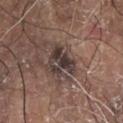Assessment: The lesion was tiled from a total-body skin photograph and was not biopsied. Background: Located on the chest. An algorithmic analysis of the crop reported a lesion area of about 8 mm², an eccentricity of roughly 0.65, and a shape-asymmetry score of about 0.45 (0 = symmetric). The analysis additionally found an average lesion color of about L≈36 a*≈12 b*≈17 (CIELAB), roughly 12 lightness units darker than nearby skin, and a normalized lesion–skin contrast near 11. The software also gave a nevus-likeness score of about 15/100. Approximately 4 mm at its widest. A male patient aged approximately 80. Cropped from a total-body skin-imaging series; the visible field is about 15 mm. The tile uses white-light illumination.